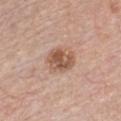* biopsy status: catalogued during a skin exam; not biopsied
* patient: male, roughly 60 years of age
* lesion size: ~3.5 mm (longest diameter)
* image: 15 mm crop, total-body photography
* anatomic site: the front of the torso
* TBP lesion metrics: a color-variation rating of about 5.5/10 and radial color variation of about 2; a lesion-detection confidence of about 100/100